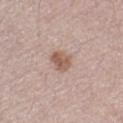Clinical impression:
The lesion was photographed on a routine skin check and not biopsied; there is no pathology result.
Acquisition and patient details:
A male patient roughly 30 years of age. The lesion-visualizer software estimated a footprint of about 5 mm², an outline eccentricity of about 0.55 (0 = round, 1 = elongated), and two-axis asymmetry of about 0.2. Approximately 2.5 mm at its widest. Captured under white-light illumination. A roughly 15 mm field-of-view crop from a total-body skin photograph.